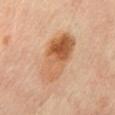notes: total-body-photography surveillance lesion; no biopsy
acquisition: 15 mm crop, total-body photography
lighting: cross-polarized illumination
patient: female, roughly 60 years of age
body site: the mid back